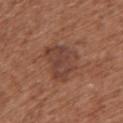Assessment:
The lesion was photographed on a routine skin check and not biopsied; there is no pathology result.
Context:
An algorithmic analysis of the crop reported a border-irregularity rating of about 4/10, internal color variation of about 3 on a 0–10 scale, and a peripheral color-asymmetry measure near 1. It also reported an automated nevus-likeness rating near 0 out of 100 and a detector confidence of about 100 out of 100 that the crop contains a lesion. Captured under white-light illumination. A female patient, in their mid- to late 70s. A close-up tile cropped from a whole-body skin photograph, about 15 mm across. The lesion is located on the upper back.